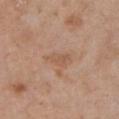workup — imaged on a skin check; not biopsied
diameter — ~3.5 mm (longest diameter)
subject — female, aged approximately 65
illumination — white-light
image-analysis metrics — a shape-asymmetry score of about 0.3 (0 = symmetric); an average lesion color of about L≈56 a*≈20 b*≈31 (CIELAB), roughly 6 lightness units darker than nearby skin, and a lesion-to-skin contrast of about 5 (normalized; higher = more distinct); border irregularity of about 3 on a 0–10 scale and a color-variation rating of about 2.5/10
imaging modality — 15 mm crop, total-body photography
body site — the chest